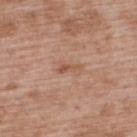The lesion's longest dimension is about 3 mm. The lesion is located on the upper back. A close-up tile cropped from a whole-body skin photograph, about 15 mm across. Imaged with white-light lighting. A male patient about 50 years old.